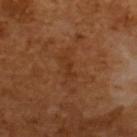Impression:
Part of a total-body skin-imaging series; this lesion was reviewed on a skin check and was not flagged for biopsy.
Clinical summary:
A region of skin cropped from a whole-body photographic capture, roughly 15 mm wide. This is a cross-polarized tile. A male patient in their mid- to late 60s. The recorded lesion diameter is about 3 mm. Automated tile analysis of the lesion measured an outline eccentricity of about 0.95 (0 = round, 1 = elongated) and a symmetry-axis asymmetry near 0.35. It also reported an average lesion color of about L≈33 a*≈22 b*≈33 (CIELAB), roughly 5 lightness units darker than nearby skin, and a normalized lesion–skin contrast near 5.5.